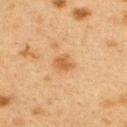Clinical impression: Part of a total-body skin-imaging series; this lesion was reviewed on a skin check and was not flagged for biopsy. Image and clinical context: About 2.5 mm across. Cropped from a whole-body photographic skin survey; the tile spans about 15 mm. Captured under cross-polarized illumination. On the upper back. The patient is a female aged 38 to 42. Automated tile analysis of the lesion measured a lesion area of about 4.5 mm², a shape eccentricity near 0.6, and a shape-asymmetry score of about 0.25 (0 = symmetric). And it measured border irregularity of about 2 on a 0–10 scale, a color-variation rating of about 2/10, and a peripheral color-asymmetry measure near 0.5.